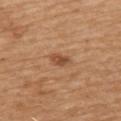No biopsy was performed on this lesion — it was imaged during a full skin examination and was not determined to be concerning. This is a white-light tile. The lesion is on the upper back. A male subject aged around 70. A 15 mm close-up tile from a total-body photography series done for melanoma screening.